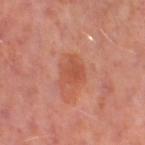Q: Was a biopsy performed?
A: total-body-photography surveillance lesion; no biopsy
Q: What kind of image is this?
A: ~15 mm tile from a whole-body skin photo
Q: What is the anatomic site?
A: the leg
Q: Patient demographics?
A: female, aged approximately 50